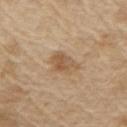Impression: Captured during whole-body skin photography for melanoma surveillance; the lesion was not biopsied. Acquisition and patient details: This is a white-light tile. The subject is a male in their 70s. From the arm. A 15 mm close-up extracted from a 3D total-body photography capture. The recorded lesion diameter is about 3.5 mm. The total-body-photography lesion software estimated an area of roughly 7.5 mm², an eccentricity of roughly 0.7, and a symmetry-axis asymmetry near 0.3. The software also gave a mean CIELAB color near L≈56 a*≈16 b*≈33 and a normalized lesion–skin contrast near 6.5.